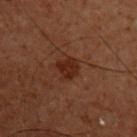No biopsy was performed on this lesion — it was imaged during a full skin examination and was not determined to be concerning. A male patient roughly 60 years of age. Captured under cross-polarized illumination. The lesion is on the chest. Cropped from a whole-body photographic skin survey; the tile spans about 15 mm. Automated tile analysis of the lesion measured a mean CIELAB color near L≈19 a*≈18 b*≈22, a lesion–skin lightness drop of about 6, and a normalized border contrast of about 8.5.